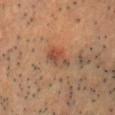A male patient, aged around 65.
Cropped from a whole-body photographic skin survey; the tile spans about 15 mm.
The lesion-visualizer software estimated a lesion color around L≈49 a*≈24 b*≈31 in CIELAB. And it measured internal color variation of about 7.5 on a 0–10 scale and peripheral color asymmetry of about 2.5.
From the head or neck.
Captured under cross-polarized illumination.